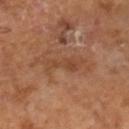Case summary:
– notes · imaged on a skin check; not biopsied
– patient · male, in their mid- to late 60s
– image · ~15 mm tile from a whole-body skin photo
– image-analysis metrics · two-axis asymmetry of about 0.4; a lesion color around L≈43 a*≈21 b*≈31 in CIELAB, roughly 7 lightness units darker than nearby skin, and a lesion-to-skin contrast of about 5.5 (normalized; higher = more distinct); a nevus-likeness score of about 0/100 and a detector confidence of about 100 out of 100 that the crop contains a lesion
– lesion diameter · ≈5 mm